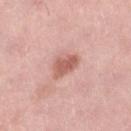Captured during whole-body skin photography for melanoma surveillance; the lesion was not biopsied. On the leg. A female patient, in their 50s. A lesion tile, about 15 mm wide, cut from a 3D total-body photograph. The recorded lesion diameter is about 3 mm.